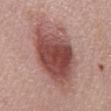Findings:
* follow-up — imaged on a skin check; not biopsied
* patient — male, roughly 70 years of age
* body site — the mid back
* tile lighting — white-light
* TBP lesion metrics — a classifier nevus-likeness of about 95/100 and a detector confidence of about 100 out of 100 that the crop contains a lesion
* imaging modality — ~15 mm crop, total-body skin-cancer survey